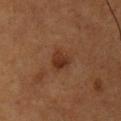{"biopsy_status": "not biopsied; imaged during a skin examination", "automated_metrics": {"area_mm2_approx": 4.5, "eccentricity": 0.45, "shape_asymmetry": 0.35, "cielab_L": 30, "cielab_a": 20, "cielab_b": 28, "vs_skin_darker_L": 8.0, "vs_skin_contrast_norm": 8.0, "border_irregularity_0_10": 3.0, "color_variation_0_10": 3.5, "nevus_likeness_0_100": 50}, "image": {"source": "total-body photography crop", "field_of_view_mm": 15}, "lesion_size": {"long_diameter_mm_approx": 2.5}, "patient": {"sex": "male", "age_approx": 55}, "site": "chest", "lighting": "cross-polarized"}